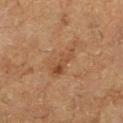Q: Automated lesion metrics?
A: a lesion area of about 8.5 mm², a shape eccentricity near 0.85, and two-axis asymmetry of about 0.4
Q: Who is the patient?
A: female, approximately 50 years of age
Q: How large is the lesion?
A: ≈4.5 mm
Q: How was this image acquired?
A: ~15 mm tile from a whole-body skin photo
Q: What lighting was used for the tile?
A: cross-polarized
Q: Lesion location?
A: the left lower leg